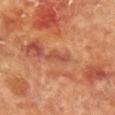Case summary:
* workup — no biopsy performed (imaged during a skin exam)
* anatomic site — the chest
* lesion size — ≈3 mm
* patient — female, aged 78 to 82
* lighting — cross-polarized
* image — total-body-photography crop, ~15 mm field of view